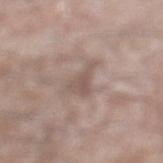Q: Is there a histopathology result?
A: total-body-photography surveillance lesion; no biopsy
Q: Where on the body is the lesion?
A: the left lower leg
Q: Automated lesion metrics?
A: an area of roughly 6.5 mm², a shape eccentricity near 0.75, and a shape-asymmetry score of about 0.55 (0 = symmetric); a mean CIELAB color near L≈54 a*≈15 b*≈22 and a normalized lesion–skin contrast near 5.5
Q: How was this image acquired?
A: 15 mm crop, total-body photography
Q: What is the lesion's diameter?
A: ≈4 mm
Q: What lighting was used for the tile?
A: white-light illumination
Q: Patient demographics?
A: male, aged approximately 75